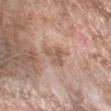Findings:
• follow-up: imaged on a skin check; not biopsied
• imaging modality: 15 mm crop, total-body photography
• body site: the right forearm
• patient: female, aged approximately 75